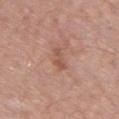Recorded during total-body skin imaging; not selected for excision or biopsy. Approximately 3 mm at its widest. This image is a 15 mm lesion crop taken from a total-body photograph. The lesion is located on the chest. A female patient, in their mid- to late 40s. Captured under white-light illumination. Automated tile analysis of the lesion measured a border-irregularity index near 4.5/10 and a within-lesion color-variation index near 0.5/10. It also reported an automated nevus-likeness rating near 0 out of 100 and lesion-presence confidence of about 100/100.